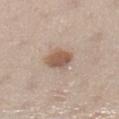Clinical impression:
This lesion was catalogued during total-body skin photography and was not selected for biopsy.
Acquisition and patient details:
Captured under white-light illumination. The patient is a female aged 38–42. Cropped from a whole-body photographic skin survey; the tile spans about 15 mm. The lesion's longest dimension is about 3 mm. Automated image analysis of the tile measured an area of roughly 7 mm². The lesion is located on the left lower leg.